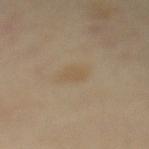| field | value |
|---|---|
| workup | imaged on a skin check; not biopsied |
| lesion size | ~2.5 mm (longest diameter) |
| patient | female, about 45 years old |
| body site | the mid back |
| image | 15 mm crop, total-body photography |
| automated lesion analysis | an automated nevus-likeness rating near 5 out of 100 and a detector confidence of about 100 out of 100 that the crop contains a lesion |
| lighting | cross-polarized illumination |